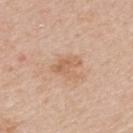follow-up: imaged on a skin check; not biopsied
image source: ~15 mm crop, total-body skin-cancer survey
illumination: white-light
location: the upper back
patient: male, approximately 65 years of age